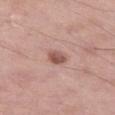Q: Was this lesion biopsied?
A: no biopsy performed (imaged during a skin exam)
Q: Illumination type?
A: white-light illumination
Q: Where on the body is the lesion?
A: the leg
Q: What are the patient's age and sex?
A: male, about 55 years old
Q: What kind of image is this?
A: total-body-photography crop, ~15 mm field of view
Q: Lesion size?
A: ~2.5 mm (longest diameter)
Q: Automated lesion metrics?
A: a lesion area of about 4 mm² and two-axis asymmetry of about 0.2; a border-irregularity index near 1.5/10, a within-lesion color-variation index near 3/10, and radial color variation of about 1; a classifier nevus-likeness of about 85/100 and lesion-presence confidence of about 100/100